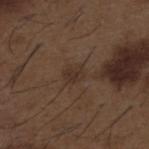The lesion was tiled from a total-body skin photograph and was not biopsied.
This is a white-light tile.
A male subject, roughly 50 years of age.
The lesion is located on the upper back.
A lesion tile, about 15 mm wide, cut from a 3D total-body photograph.
An algorithmic analysis of the crop reported an automated nevus-likeness rating near 0 out of 100.
Longest diameter approximately 2.5 mm.